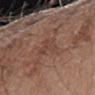Part of a total-body skin-imaging series; this lesion was reviewed on a skin check and was not flagged for biopsy.